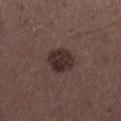Clinical impression:
Part of a total-body skin-imaging series; this lesion was reviewed on a skin check and was not flagged for biopsy.
Clinical summary:
The subject is a male in their mid-50s. Imaged with white-light lighting. About 3.5 mm across. A close-up tile cropped from a whole-body skin photograph, about 15 mm across. On the leg.